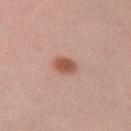Approximately 2.5 mm at its widest. A 15 mm close-up extracted from a 3D total-body photography capture. Located on the right upper arm. The subject is a female aged 23 to 27.